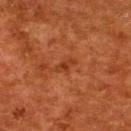notes = no biopsy performed (imaged during a skin exam) | acquisition = total-body-photography crop, ~15 mm field of view | patient = female, aged 48 to 52 | lesion size = ≈2.5 mm | location = the upper back.